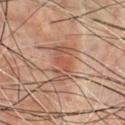No biopsy was performed on this lesion — it was imaged during a full skin examination and was not determined to be concerning. The recorded lesion diameter is about 4.5 mm. A male patient, aged around 70. The lesion is on the chest. Cropped from a total-body skin-imaging series; the visible field is about 15 mm.